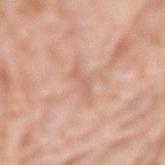| field | value |
|---|---|
| biopsy status | no biopsy performed (imaged during a skin exam) |
| image source | ~15 mm crop, total-body skin-cancer survey |
| patient | male, aged 78–82 |
| body site | the right forearm |
| tile lighting | white-light illumination |
| lesion diameter | ~4.5 mm (longest diameter) |